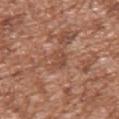workup = imaged on a skin check; not biopsied.